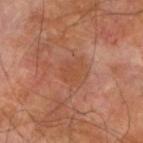Part of a total-body skin-imaging series; this lesion was reviewed on a skin check and was not flagged for biopsy. A lesion tile, about 15 mm wide, cut from a 3D total-body photograph. This is a cross-polarized tile. The lesion-visualizer software estimated a border-irregularity index near 4.5/10, internal color variation of about 1 on a 0–10 scale, and a peripheral color-asymmetry measure near 0.5. The analysis additionally found a nevus-likeness score of about 5/100 and a lesion-detection confidence of about 100/100. From the arm. The lesion's longest dimension is about 3 mm. The subject is a male about 70 years old.